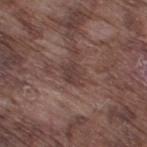The lesion was tiled from a total-body skin photograph and was not biopsied. A male subject, approximately 75 years of age. The lesion's longest dimension is about 3 mm. From the leg. Cropped from a total-body skin-imaging series; the visible field is about 15 mm.